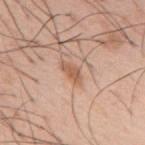No biopsy was performed on this lesion — it was imaged during a full skin examination and was not determined to be concerning.
A close-up tile cropped from a whole-body skin photograph, about 15 mm across.
The lesion is located on the mid back.
The patient is a male aged around 55.